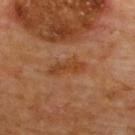Assessment:
This lesion was catalogued during total-body skin photography and was not selected for biopsy.
Context:
Captured under cross-polarized illumination. The subject is a male in their 60s. The lesion's longest dimension is about 4.5 mm. A 15 mm close-up tile from a total-body photography series done for melanoma screening. From the back.